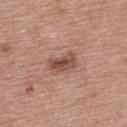No biopsy was performed on this lesion — it was imaged during a full skin examination and was not determined to be concerning.
Automated image analysis of the tile measured a lesion area of about 5.5 mm², a shape eccentricity near 0.85, and two-axis asymmetry of about 0.25. The analysis additionally found a color-variation rating of about 4/10 and a peripheral color-asymmetry measure near 1.5.
A lesion tile, about 15 mm wide, cut from a 3D total-body photograph.
The tile uses white-light illumination.
The recorded lesion diameter is about 3.5 mm.
The patient is a female aged approximately 50.
The lesion is located on the upper back.